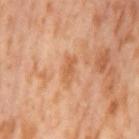* workup · catalogued during a skin exam; not biopsied
* lesion diameter · ~3 mm (longest diameter)
* acquisition · ~15 mm crop, total-body skin-cancer survey
* patient · female, aged 53 to 57
* automated metrics · a footprint of about 3.5 mm², an outline eccentricity of about 0.9 (0 = round, 1 = elongated), and two-axis asymmetry of about 0.3; internal color variation of about 0.5 on a 0–10 scale and a peripheral color-asymmetry measure near 0
* site · the left thigh